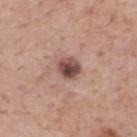- workup: imaged on a skin check; not biopsied
- body site: the mid back
- lighting: white-light
- image-analysis metrics: a mean CIELAB color near L≈48 a*≈20 b*≈22, a lesion–skin lightness drop of about 15, and a lesion-to-skin contrast of about 11 (normalized; higher = more distinct); a border-irregularity rating of about 1.5/10, a color-variation rating of about 6.5/10, and radial color variation of about 2
- patient: male, aged 73–77
- lesion diameter: about 3 mm
- image source: total-body-photography crop, ~15 mm field of view